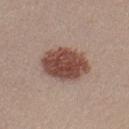automated metrics: an outline eccentricity of about 0.6 (0 = round, 1 = elongated) and a shape-asymmetry score of about 0.1 (0 = symmetric); a mean CIELAB color near L≈47 a*≈21 b*≈24 and roughly 16 lightness units darker than nearby skin; an automated nevus-likeness rating near 100 out of 100
lesion diameter: ≈5.5 mm
image source: 15 mm crop, total-body photography
location: the left upper arm
subject: female, aged 28–32